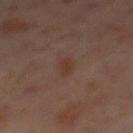{
  "biopsy_status": "not biopsied; imaged during a skin examination",
  "patient": {
    "sex": "male",
    "age_approx": 60
  },
  "site": "mid back",
  "image": {
    "source": "total-body photography crop",
    "field_of_view_mm": 15
  }
}